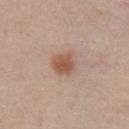<tbp_lesion>
<image>
  <source>total-body photography crop</source>
  <field_of_view_mm>15</field_of_view_mm>
</image>
<site>chest</site>
<lesion_size>
  <long_diameter_mm_approx>3.0</long_diameter_mm_approx>
</lesion_size>
<patient>
  <sex>male</sex>
  <age_approx>55</age_approx>
</patient>
</tbp_lesion>